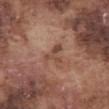Context:
A 15 mm crop from a total-body photograph taken for skin-cancer surveillance. On the abdomen. A male subject in their mid- to late 70s.